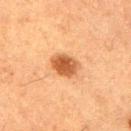notes=catalogued during a skin exam; not biopsied
automated metrics=a footprint of about 7 mm² and a symmetry-axis asymmetry near 0.2; internal color variation of about 4 on a 0–10 scale and peripheral color asymmetry of about 1; a classifier nevus-likeness of about 100/100
image source=15 mm crop, total-body photography
tile lighting=cross-polarized illumination
patient=male, about 50 years old
lesion size=~3.5 mm (longest diameter)
body site=the right upper arm